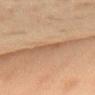Context:
A lesion tile, about 15 mm wide, cut from a 3D total-body photograph. From the upper back. A female patient, about 60 years old.